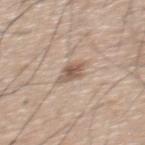  biopsy_status: not biopsied; imaged during a skin examination
  site: upper back
  lighting: white-light
  lesion_size:
    long_diameter_mm_approx: 3.0
  patient:
    sex: male
    age_approx: 65
  image:
    source: total-body photography crop
    field_of_view_mm: 15